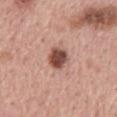Findings:
• patient: male, aged around 60
• image source: 15 mm crop, total-body photography
• site: the mid back
• lesion diameter: ~3 mm (longest diameter)
• illumination: white-light illumination